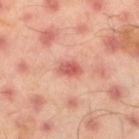Impression: Part of a total-body skin-imaging series; this lesion was reviewed on a skin check and was not flagged for biopsy.